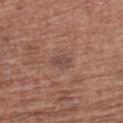Acquisition and patient details: A 15 mm close-up tile from a total-body photography series done for melanoma screening. The lesion is on the upper back. Approximately 2.5 mm at its widest. Captured under white-light illumination. A female subject roughly 65 years of age. An algorithmic analysis of the crop reported an automated nevus-likeness rating near 5 out of 100 and lesion-presence confidence of about 100/100.